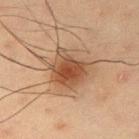This lesion was catalogued during total-body skin photography and was not selected for biopsy. An algorithmic analysis of the crop reported an area of roughly 20 mm², an eccentricity of roughly 0.55, and a shape-asymmetry score of about 0.25 (0 = symmetric). The software also gave a lesion color around L≈47 a*≈18 b*≈30 in CIELAB, roughly 11 lightness units darker than nearby skin, and a lesion-to-skin contrast of about 8 (normalized; higher = more distinct). The software also gave a border-irregularity index near 4/10 and a within-lesion color-variation index near 6.5/10. The analysis additionally found a classifier nevus-likeness of about 95/100 and lesion-presence confidence of about 100/100. A male patient in their mid-30s. The lesion is on the right upper arm. A close-up tile cropped from a whole-body skin photograph, about 15 mm across. Measured at roughly 6 mm in maximum diameter. The tile uses cross-polarized illumination.